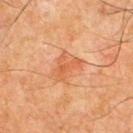- follow-up — total-body-photography surveillance lesion; no biopsy
- body site — the chest
- patient — male, roughly 65 years of age
- image — ~15 mm tile from a whole-body skin photo
- TBP lesion metrics — a lesion–skin lightness drop of about 7; a nevus-likeness score of about 0/100